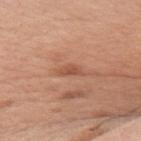Recorded during total-body skin imaging; not selected for excision or biopsy.
Approximately 3 mm at its widest.
On the right upper arm.
A female patient aged 48 to 52.
Cropped from a total-body skin-imaging series; the visible field is about 15 mm.
This is a white-light tile.